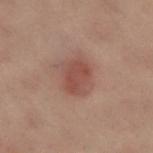Imaged during a routine full-body skin examination; the lesion was not biopsied and no histopathology is available.
About 3.5 mm across.
Imaged with cross-polarized lighting.
A female subject aged around 60.
On the leg.
A 15 mm close-up tile from a total-body photography series done for melanoma screening.